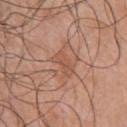Assessment: Imaged during a routine full-body skin examination; the lesion was not biopsied and no histopathology is available. Acquisition and patient details: The subject is a male aged 68 to 72. From the front of the torso. This image is a 15 mm lesion crop taken from a total-body photograph.